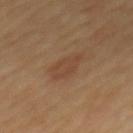Captured during whole-body skin photography for melanoma surveillance; the lesion was not biopsied.
On the mid back.
Longest diameter approximately 4 mm.
A 15 mm crop from a total-body photograph taken for skin-cancer surveillance.
The patient is a male in their 60s.
Captured under cross-polarized illumination.